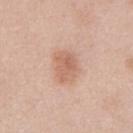Notes:
* follow-up — total-body-photography surveillance lesion; no biopsy
* subject — female, about 40 years old
* image source — ~15 mm crop, total-body skin-cancer survey
* lighting — white-light illumination
* automated lesion analysis — a mean CIELAB color near L≈63 a*≈21 b*≈30, a lesion–skin lightness drop of about 9, and a normalized lesion–skin contrast near 6.5; a nevus-likeness score of about 75/100
* location — the mid back
* lesion diameter — ≈3.5 mm